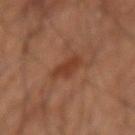Assessment:
Recorded during total-body skin imaging; not selected for excision or biopsy.
Acquisition and patient details:
A male patient, about 55 years old. This image is a 15 mm lesion crop taken from a total-body photograph. The recorded lesion diameter is about 3 mm. The total-body-photography lesion software estimated roughly 8 lightness units darker than nearby skin. The tile uses cross-polarized illumination. On the right forearm.